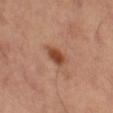workup — imaged on a skin check; not biopsied
subject — male, aged 63 to 67
lesion size — ~3.5 mm (longest diameter)
image — ~15 mm crop, total-body skin-cancer survey
TBP lesion metrics — a footprint of about 5 mm², an eccentricity of roughly 0.85, and two-axis asymmetry of about 0.3
illumination — cross-polarized illumination